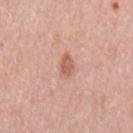biopsy_status: not biopsied; imaged during a skin examination
site: mid back
patient:
  sex: male
  age_approx: 60
image:
  source: total-body photography crop
  field_of_view_mm: 15